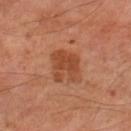{"biopsy_status": "not biopsied; imaged during a skin examination", "image": {"source": "total-body photography crop", "field_of_view_mm": 15}, "site": "left lower leg", "lesion_size": {"long_diameter_mm_approx": 3.5}, "lighting": "cross-polarized", "patient": {"sex": "male", "age_approx": 70}}